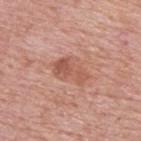| feature | finding |
|---|---|
| notes | imaged on a skin check; not biopsied |
| patient | male, approximately 75 years of age |
| diameter | ≈4.5 mm |
| tile lighting | white-light |
| automated metrics | an outline eccentricity of about 0.8 (0 = round, 1 = elongated) and a symmetry-axis asymmetry near 0.3; a mean CIELAB color near L≈56 a*≈24 b*≈29 and a lesion–skin lightness drop of about 9; border irregularity of about 4 on a 0–10 scale, internal color variation of about 5 on a 0–10 scale, and peripheral color asymmetry of about 2 |
| anatomic site | the upper back |
| image | total-body-photography crop, ~15 mm field of view |